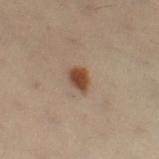workup: imaged on a skin check; not biopsied | body site: the right thigh | automated metrics: about 11 CIELAB-L* units darker than the surrounding skin and a normalized border contrast of about 10.5; a classifier nevus-likeness of about 100/100 and lesion-presence confidence of about 100/100 | diameter: ~3 mm (longest diameter) | acquisition: total-body-photography crop, ~15 mm field of view | patient: female, in their 50s.